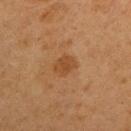Imaged during a routine full-body skin examination; the lesion was not biopsied and no histopathology is available. A close-up tile cropped from a whole-body skin photograph, about 15 mm across. The lesion is on the left upper arm. Captured under cross-polarized illumination. The subject is a female approximately 40 years of age.